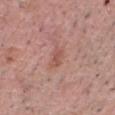| key | value |
|---|---|
| follow-up | imaged on a skin check; not biopsied |
| site | the head or neck |
| illumination | white-light illumination |
| diameter | ~2.5 mm (longest diameter) |
| patient | male, aged 53–57 |
| automated metrics | internal color variation of about 0.5 on a 0–10 scale and a peripheral color-asymmetry measure near 0 |
| acquisition | ~15 mm tile from a whole-body skin photo |